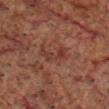{
  "biopsy_status": "not biopsied; imaged during a skin examination",
  "patient": {
    "sex": "male",
    "age_approx": 60
  },
  "site": "head or neck",
  "image": {
    "source": "total-body photography crop",
    "field_of_view_mm": 15
  }
}